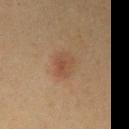Clinical impression: Recorded during total-body skin imaging; not selected for excision or biopsy. Background: Approximately 3 mm at its widest. From the right upper arm. Imaged with cross-polarized lighting. Automated tile analysis of the lesion measured a lesion area of about 6 mm², a shape eccentricity near 0.65, and two-axis asymmetry of about 0.2. The analysis additionally found a lesion color around L≈40 a*≈17 b*≈27 in CIELAB, roughly 6 lightness units darker than nearby skin, and a normalized lesion–skin contrast near 5.5. And it measured a classifier nevus-likeness of about 95/100 and lesion-presence confidence of about 100/100. A lesion tile, about 15 mm wide, cut from a 3D total-body photograph. A female subject, roughly 40 years of age.